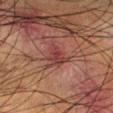The lesion was photographed on a routine skin check and not biopsied; there is no pathology result. The lesion is located on the right lower leg. Longest diameter approximately 2.5 mm. Cropped from a total-body skin-imaging series; the visible field is about 15 mm. Captured under cross-polarized illumination. The patient is a male roughly 50 years of age.